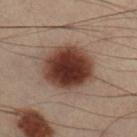biopsy status: catalogued during a skin exam; not biopsied
body site: the right lower leg
lesion size: ≈6 mm
automated lesion analysis: a nevus-likeness score of about 100/100 and a lesion-detection confidence of about 100/100
subject: male, aged 53–57
illumination: cross-polarized
acquisition: ~15 mm crop, total-body skin-cancer survey